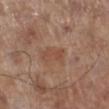<lesion>
  <biopsy_status>not biopsied; imaged during a skin examination</biopsy_status>
  <patient>
    <sex>male</sex>
    <age_approx>70</age_approx>
  </patient>
  <lesion_size>
    <long_diameter_mm_approx>3.0</long_diameter_mm_approx>
  </lesion_size>
  <image>
    <source>total-body photography crop</source>
    <field_of_view_mm>15</field_of_view_mm>
  </image>
  <lighting>white-light</lighting>
  <site>left lower leg</site>
</lesion>